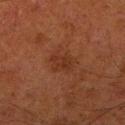No biopsy was performed on this lesion — it was imaged during a full skin examination and was not determined to be concerning. The patient is a male about 80 years old. Captured under cross-polarized illumination. About 3 mm across. The lesion is on the right lower leg. A region of skin cropped from a whole-body photographic capture, roughly 15 mm wide.